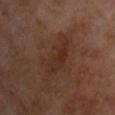Impression: Part of a total-body skin-imaging series; this lesion was reviewed on a skin check and was not flagged for biopsy. Clinical summary: The subject is a male aged 53 to 57. On the back. A close-up tile cropped from a whole-body skin photograph, about 15 mm across.